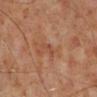The lesion was tiled from a total-body skin photograph and was not biopsied.
The lesion is on the right lower leg.
Automated tile analysis of the lesion measured a border-irregularity index near 5.5/10, a within-lesion color-variation index near 0/10, and a peripheral color-asymmetry measure near 0. It also reported a lesion-detection confidence of about 100/100.
Imaged with cross-polarized lighting.
A region of skin cropped from a whole-body photographic capture, roughly 15 mm wide.
A male subject roughly 70 years of age.